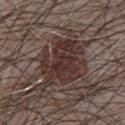The lesion was photographed on a routine skin check and not biopsied; there is no pathology result. A male subject, aged around 65. A lesion tile, about 15 mm wide, cut from a 3D total-body photograph. The lesion is on the chest.